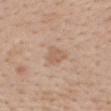{
  "biopsy_status": "not biopsied; imaged during a skin examination",
  "lesion_size": {
    "long_diameter_mm_approx": 2.5
  },
  "site": "back",
  "image": {
    "source": "total-body photography crop",
    "field_of_view_mm": 15
  },
  "lighting": "white-light",
  "patient": {
    "sex": "female",
    "age_approx": 55
  }
}